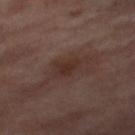Q: Is there a histopathology result?
A: imaged on a skin check; not biopsied
Q: Illumination type?
A: cross-polarized illumination
Q: What kind of image is this?
A: 15 mm crop, total-body photography
Q: What is the lesion's diameter?
A: about 3 mm
Q: What did automated image analysis measure?
A: a border-irregularity index near 3.5/10, a color-variation rating of about 1/10, and a peripheral color-asymmetry measure near 0.5
Q: Where on the body is the lesion?
A: the right thigh
Q: Who is the patient?
A: female, in their mid- to late 50s